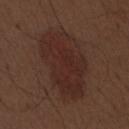Imaged during a routine full-body skin examination; the lesion was not biopsied and no histopathology is available.
This is a white-light tile.
A 15 mm close-up tile from a total-body photography series done for melanoma screening.
The lesion's longest dimension is about 9 mm.
A male patient aged approximately 70.
The lesion is located on the abdomen.